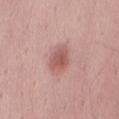Recorded during total-body skin imaging; not selected for excision or biopsy.
From the front of the torso.
Imaged with white-light lighting.
A close-up tile cropped from a whole-body skin photograph, about 15 mm across.
A male subject aged approximately 60.
The lesion's longest dimension is about 3.5 mm.